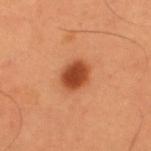This lesion was catalogued during total-body skin photography and was not selected for biopsy. The patient is a male approximately 55 years of age. A close-up tile cropped from a whole-body skin photograph, about 15 mm across. The lesion's longest dimension is about 3.5 mm. On the back.